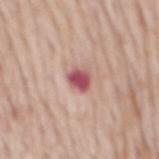This lesion was catalogued during total-body skin photography and was not selected for biopsy.
Captured under white-light illumination.
The lesion is located on the mid back.
A male subject about 65 years old.
A 15 mm close-up tile from a total-body photography series done for melanoma screening.
Measured at roughly 2.5 mm in maximum diameter.